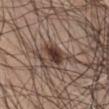Part of a total-body skin-imaging series; this lesion was reviewed on a skin check and was not flagged for biopsy. Measured at roughly 3 mm in maximum diameter. The lesion is located on the abdomen. The patient is a male about 70 years old. A 15 mm close-up tile from a total-body photography series done for melanoma screening.